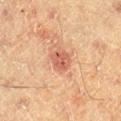Assessment: Part of a total-body skin-imaging series; this lesion was reviewed on a skin check and was not flagged for biopsy. Clinical summary: A male patient, aged approximately 60. A 15 mm crop from a total-body photograph taken for skin-cancer surveillance. Captured under cross-polarized illumination. Located on the right lower leg. The recorded lesion diameter is about 3 mm.